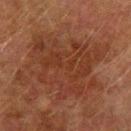{
  "lesion_size": {
    "long_diameter_mm_approx": 8.5
  },
  "site": "left upper arm",
  "lighting": "cross-polarized",
  "automated_metrics": {
    "border_irregularity_0_10": 7.5,
    "color_variation_0_10": 3.5,
    "peripheral_color_asymmetry": 1.5,
    "lesion_detection_confidence_0_100": 100
  },
  "patient": {
    "sex": "male",
    "age_approx": 75
  },
  "image": {
    "source": "total-body photography crop",
    "field_of_view_mm": 15
  }
}